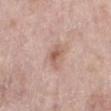This lesion was catalogued during total-body skin photography and was not selected for biopsy. The tile uses white-light illumination. The patient is a female approximately 70 years of age. Approximately 2.5 mm at its widest. A 15 mm close-up tile from a total-body photography series done for melanoma screening. The lesion is on the leg.